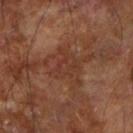notes = catalogued during a skin exam; not biopsied | subject = male, in their mid- to late 60s | automated lesion analysis = a classifier nevus-likeness of about 0/100 and lesion-presence confidence of about 60/100 | acquisition = 15 mm crop, total-body photography | anatomic site = the right forearm | lesion size = about 4.5 mm.